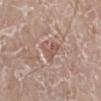Clinical impression: The lesion was photographed on a routine skin check and not biopsied; there is no pathology result. Image and clinical context: A male patient, aged approximately 80. From the right leg. This image is a 15 mm lesion crop taken from a total-body photograph.